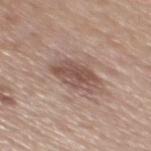Measured at roughly 4.5 mm in maximum diameter.
Imaged with white-light lighting.
An algorithmic analysis of the crop reported a border-irregularity rating of about 2.5/10, internal color variation of about 4 on a 0–10 scale, and radial color variation of about 1.5.
This image is a 15 mm lesion crop taken from a total-body photograph.
A male patient, about 50 years old.
The lesion is located on the mid back.